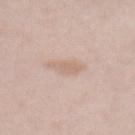biopsy status = total-body-photography surveillance lesion; no biopsy | imaging modality = total-body-photography crop, ~15 mm field of view | size = ~3 mm (longest diameter) | subject = female, approximately 40 years of age | anatomic site = the abdomen | tile lighting = white-light.